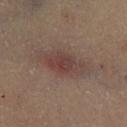  biopsy_status: not biopsied; imaged during a skin examination
  image:
    source: total-body photography crop
    field_of_view_mm: 15
  lesion_size:
    long_diameter_mm_approx: 6.5
  automated_metrics:
    area_mm2_approx: 15.0
    eccentricity: 0.85
    nevus_likeness_0_100: 30
    lesion_detection_confidence_0_100: 100
  lighting: cross-polarized
  patient:
    sex: male
    age_approx: 60